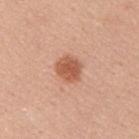biopsy status: total-body-photography surveillance lesion; no biopsy
size: ≈3.5 mm
subject: male, in their mid- to late 30s
imaging modality: ~15 mm tile from a whole-body skin photo
location: the upper back